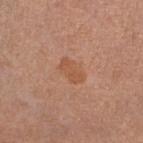biopsy status: imaged on a skin check; not biopsied | lighting: white-light | diameter: ~3 mm (longest diameter) | image: ~15 mm crop, total-body skin-cancer survey | patient: female, roughly 55 years of age | site: the left lower leg.